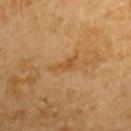{
  "biopsy_status": "not biopsied; imaged during a skin examination",
  "image": {
    "source": "total-body photography crop",
    "field_of_view_mm": 15
  },
  "patient": {
    "sex": "male",
    "age_approx": "85+"
  },
  "site": "back"
}